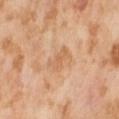<case>
  <patient>
    <sex>female</sex>
    <age_approx>55</age_approx>
  </patient>
  <lesion_size>
    <long_diameter_mm_approx>3.5</long_diameter_mm_approx>
  </lesion_size>
  <image>
    <source>total-body photography crop</source>
    <field_of_view_mm>15</field_of_view_mm>
  </image>
  <lighting>cross-polarized</lighting>
  <site>front of the torso</site>
</case>